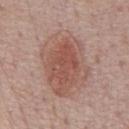biopsy status: total-body-photography surveillance lesion; no biopsy | subject: male, aged approximately 75 | body site: the chest | acquisition: 15 mm crop, total-body photography.